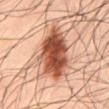Clinical impression:
No biopsy was performed on this lesion — it was imaged during a full skin examination and was not determined to be concerning.
Background:
Imaged with cross-polarized lighting. A roughly 15 mm field-of-view crop from a total-body skin photograph. Measured at roughly 8.5 mm in maximum diameter. An algorithmic analysis of the crop reported an average lesion color of about L≈52 a*≈27 b*≈33 (CIELAB), roughly 21 lightness units darker than nearby skin, and a lesion-to-skin contrast of about 13 (normalized; higher = more distinct). And it measured a border-irregularity index near 3.5/10 and radial color variation of about 3. And it measured a nevus-likeness score of about 100/100 and a lesion-detection confidence of about 100/100. On the back. The subject is a male roughly 50 years of age.